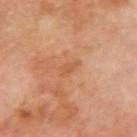biopsy status: imaged on a skin check; not biopsied | automated lesion analysis: an automated nevus-likeness rating near 0 out of 100 and a lesion-detection confidence of about 100/100 | location: the right upper arm | acquisition: ~15 mm crop, total-body skin-cancer survey | patient: male, aged 68–72.